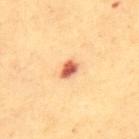No biopsy was performed on this lesion — it was imaged during a full skin examination and was not determined to be concerning. Longest diameter approximately 2.5 mm. The tile uses cross-polarized illumination. Cropped from a total-body skin-imaging series; the visible field is about 15 mm. The lesion is located on the upper back. A female patient aged approximately 55.